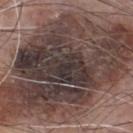Captured during whole-body skin photography for melanoma surveillance; the lesion was not biopsied.
About 18.5 mm across.
Cropped from a total-body skin-imaging series; the visible field is about 15 mm.
Located on the chest.
Automated image analysis of the tile measured an area of roughly 130 mm², an eccentricity of roughly 0.85, and a symmetry-axis asymmetry near 0.25. It also reported an automated nevus-likeness rating near 0 out of 100 and lesion-presence confidence of about 100/100.
A male subject aged 73 to 77.